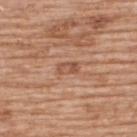Clinical impression:
Captured during whole-body skin photography for melanoma surveillance; the lesion was not biopsied.
Acquisition and patient details:
This is a white-light tile. Approximately 2.5 mm at its widest. Cropped from a whole-body photographic skin survey; the tile spans about 15 mm. From the upper back. A male patient aged approximately 60. The lesion-visualizer software estimated an average lesion color of about L≈53 a*≈23 b*≈31 (CIELAB), about 9 CIELAB-L* units darker than the surrounding skin, and a normalized border contrast of about 6. The analysis additionally found an automated nevus-likeness rating near 0 out of 100.